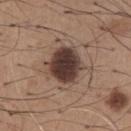Q: Lesion location?
A: the chest
Q: Who is the patient?
A: male, aged approximately 65
Q: How was this image acquired?
A: ~15 mm tile from a whole-body skin photo
Q: How large is the lesion?
A: about 4.5 mm